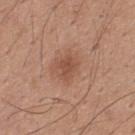Part of a total-body skin-imaging series; this lesion was reviewed on a skin check and was not flagged for biopsy. This is a white-light tile. A lesion tile, about 15 mm wide, cut from a 3D total-body photograph. The lesion's longest dimension is about 3 mm. Automated image analysis of the tile measured an area of roughly 5 mm² and a shape-asymmetry score of about 0.3 (0 = symmetric). The analysis additionally found a mean CIELAB color near L≈50 a*≈22 b*≈30, a lesion–skin lightness drop of about 8, and a normalized border contrast of about 6.5. The software also gave an automated nevus-likeness rating near 45 out of 100 and a lesion-detection confidence of about 100/100. A male patient aged 28 to 32. The lesion is on the upper back.